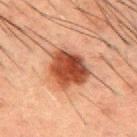{"biopsy_status": "not biopsied; imaged during a skin examination", "lighting": "cross-polarized", "site": "mid back", "image": {"source": "total-body photography crop", "field_of_view_mm": 15}, "patient": {"sex": "male", "age_approx": 50}}